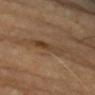Clinical impression: Part of a total-body skin-imaging series; this lesion was reviewed on a skin check and was not flagged for biopsy. Acquisition and patient details: A female subject, aged 68–72. A close-up tile cropped from a whole-body skin photograph, about 15 mm across. The lesion is on the right forearm.